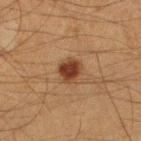Q: Is there a histopathology result?
A: catalogued during a skin exam; not biopsied
Q: How large is the lesion?
A: ~3 mm (longest diameter)
Q: Lesion location?
A: the right lower leg
Q: What did automated image analysis measure?
A: a lesion area of about 5 mm², an eccentricity of roughly 0.5, and two-axis asymmetry of about 0.3; a detector confidence of about 100 out of 100 that the crop contains a lesion
Q: How was the tile lit?
A: cross-polarized illumination
Q: Who is the patient?
A: male, aged 48 to 52
Q: How was this image acquired?
A: total-body-photography crop, ~15 mm field of view